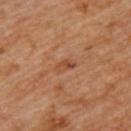biopsy status: catalogued during a skin exam; not biopsied
image-analysis metrics: an eccentricity of roughly 0.85; a mean CIELAB color near L≈46 a*≈24 b*≈33 and roughly 8 lightness units darker than nearby skin
anatomic site: the upper back
imaging modality: ~15 mm crop, total-body skin-cancer survey
size: about 2.5 mm
lighting: cross-polarized illumination
subject: male, approximately 60 years of age